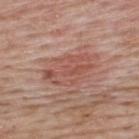This lesion was catalogued during total-body skin photography and was not selected for biopsy. The tile uses white-light illumination. The lesion's longest dimension is about 6 mm. A lesion tile, about 15 mm wide, cut from a 3D total-body photograph. Located on the upper back. A male patient in their mid- to late 60s.